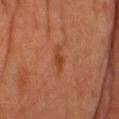Case summary:
* workup — catalogued during a skin exam; not biopsied
* patient — female, about 55 years old
* tile lighting — cross-polarized illumination
* anatomic site — the chest
* lesion size — about 3.5 mm
* acquisition — total-body-photography crop, ~15 mm field of view
* automated metrics — a footprint of about 3.5 mm², an outline eccentricity of about 0.9 (0 = round, 1 = elongated), and a shape-asymmetry score of about 0.25 (0 = symmetric); an automated nevus-likeness rating near 10 out of 100 and a detector confidence of about 100 out of 100 that the crop contains a lesion